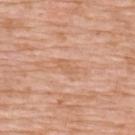| field | value |
|---|---|
| notes | no biopsy performed (imaged during a skin exam) |
| subject | female, in their 70s |
| image source | 15 mm crop, total-body photography |
| size | about 2.5 mm |
| location | the upper back |